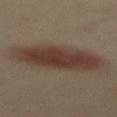patient — female, aged 38 to 42
lighting — cross-polarized
body site — the mid back
image — 15 mm crop, total-body photography
diameter — ~9 mm (longest diameter)